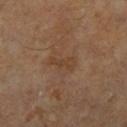Captured during whole-body skin photography for melanoma surveillance; the lesion was not biopsied. Imaged with cross-polarized lighting. A male patient aged 63 to 67. Measured at roughly 3 mm in maximum diameter. The total-body-photography lesion software estimated about 5 CIELAB-L* units darker than the surrounding skin and a normalized lesion–skin contrast near 5.5. The software also gave border irregularity of about 4.5 on a 0–10 scale, a color-variation rating of about 0.5/10, and peripheral color asymmetry of about 0. Cropped from a total-body skin-imaging series; the visible field is about 15 mm.